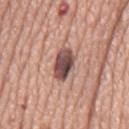Q: How was this image acquired?
A: 15 mm crop, total-body photography
Q: Who is the patient?
A: male, approximately 75 years of age
Q: What is the lesion's diameter?
A: ~4.5 mm (longest diameter)
Q: Lesion location?
A: the mid back